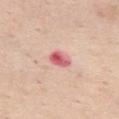The patient is a female roughly 55 years of age. Imaged with white-light lighting. Located on the left thigh. The total-body-photography lesion software estimated a shape eccentricity near 0.75 and two-axis asymmetry of about 0.25. And it measured about 15 CIELAB-L* units darker than the surrounding skin and a normalized lesion–skin contrast near 9.5. The software also gave a nevus-likeness score of about 0/100 and a detector confidence of about 100 out of 100 that the crop contains a lesion. This image is a 15 mm lesion crop taken from a total-body photograph.